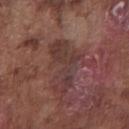Impression: Captured during whole-body skin photography for melanoma surveillance; the lesion was not biopsied. Clinical summary: The patient is a male about 75 years old. Captured under white-light illumination. Approximately 6.5 mm at its widest. Cropped from a whole-body photographic skin survey; the tile spans about 15 mm. Located on the chest.